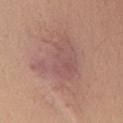No biopsy was performed on this lesion — it was imaged during a full skin examination and was not determined to be concerning. Located on the chest. A male patient aged 33 to 37. A lesion tile, about 15 mm wide, cut from a 3D total-body photograph. The recorded lesion diameter is about 6 mm.